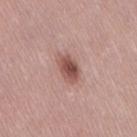Imaged during a routine full-body skin examination; the lesion was not biopsied and no histopathology is available. Longest diameter approximately 3.5 mm. The lesion is on the leg. A close-up tile cropped from a whole-body skin photograph, about 15 mm across. This is a white-light tile. The subject is a female about 40 years old. The total-body-photography lesion software estimated an area of roughly 6.5 mm², a shape eccentricity near 0.75, and a shape-asymmetry score of about 0.15 (0 = symmetric).